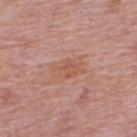Clinical impression: The lesion was photographed on a routine skin check and not biopsied; there is no pathology result. Background: Imaged with white-light lighting. On the back. The lesion's longest dimension is about 3.5 mm. A male patient aged around 75. The lesion-visualizer software estimated an outline eccentricity of about 0.85 (0 = round, 1 = elongated) and a shape-asymmetry score of about 0.3 (0 = symmetric). The software also gave a lesion–skin lightness drop of about 6 and a lesion-to-skin contrast of about 5.5 (normalized; higher = more distinct). The analysis additionally found a border-irregularity rating of about 3/10, a within-lesion color-variation index near 2.5/10, and a peripheral color-asymmetry measure near 1. A 15 mm close-up tile from a total-body photography series done for melanoma screening.